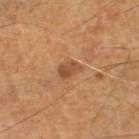| feature | finding |
|---|---|
| notes | catalogued during a skin exam; not biopsied |
| lesion size | about 2.5 mm |
| image | ~15 mm tile from a whole-body skin photo |
| anatomic site | the right lower leg |
| patient | male, aged around 45 |
| TBP lesion metrics | an average lesion color of about L≈49 a*≈22 b*≈35 (CIELAB) and a normalized lesion–skin contrast near 7 |